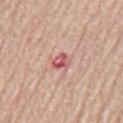Imaged during a routine full-body skin examination; the lesion was not biopsied and no histopathology is available. A roughly 15 mm field-of-view crop from a total-body skin photograph. On the chest. About 2.5 mm across. Automated tile analysis of the lesion measured a color-variation rating of about 7.5/10 and radial color variation of about 3. The patient is a male aged approximately 80.